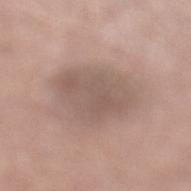follow-up: catalogued during a skin exam; not biopsied
image: ~15 mm crop, total-body skin-cancer survey
patient: male, aged 73–77
automated metrics: a footprint of about 22 mm², an eccentricity of roughly 0.5, and a symmetry-axis asymmetry near 0.25; a mean CIELAB color near L≈55 a*≈15 b*≈23 and roughly 8 lightness units darker than nearby skin
tile lighting: white-light
site: the left lower leg
lesion diameter: about 5.5 mm